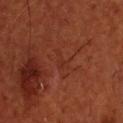<lesion>
  <biopsy_status>not biopsied; imaged during a skin examination</biopsy_status>
  <patient>
    <sex>male</sex>
    <age_approx>55</age_approx>
  </patient>
  <image>
    <source>total-body photography crop</source>
    <field_of_view_mm>15</field_of_view_mm>
  </image>
  <lesion_size>
    <long_diameter_mm_approx>3.0</long_diameter_mm_approx>
  </lesion_size>
  <site>head or neck</site>
  <automated_metrics>
    <area_mm2_approx>3.0</area_mm2_approx>
    <shape_asymmetry>0.6</shape_asymmetry>
    <cielab_L>29</cielab_L>
    <cielab_a>25</cielab_a>
    <cielab_b>28</cielab_b>
    <vs_skin_darker_L>3.0</vs_skin_darker_L>
    <border_irregularity_0_10>6.5</border_irregularity_0_10>
    <peripheral_color_asymmetry>0.0</peripheral_color_asymmetry>
  </automated_metrics>
</lesion>